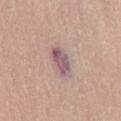workup: total-body-photography surveillance lesion; no biopsy